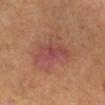Imaged during a routine full-body skin examination; the lesion was not biopsied and no histopathology is available.
A 15 mm close-up extracted from a 3D total-body photography capture.
A male subject.
The lesion is on the right lower leg.
Captured under cross-polarized illumination.
The lesion's longest dimension is about 5.5 mm.